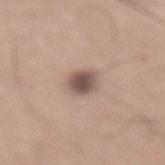Clinical impression:
The lesion was photographed on a routine skin check and not biopsied; there is no pathology result.
Acquisition and patient details:
A 15 mm close-up tile from a total-body photography series done for melanoma screening. The tile uses white-light illumination. Located on the lower back. Automated image analysis of the tile measured a lesion area of about 6 mm², an eccentricity of roughly 0.45, and a symmetry-axis asymmetry near 0.2. The analysis additionally found a lesion color around L≈52 a*≈16 b*≈22 in CIELAB, about 14 CIELAB-L* units darker than the surrounding skin, and a normalized lesion–skin contrast near 9.5. It also reported a nevus-likeness score of about 85/100 and a detector confidence of about 100 out of 100 that the crop contains a lesion. Longest diameter approximately 2.5 mm. The patient is a male in their mid-60s.